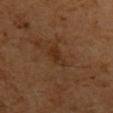The lesion was tiled from a total-body skin photograph and was not biopsied.
A 15 mm close-up tile from a total-body photography series done for melanoma screening.
The lesion is located on the arm.
A male patient, aged approximately 60.
About 3 mm across.
An algorithmic analysis of the crop reported a footprint of about 4.5 mm² and a symmetry-axis asymmetry near 0.35. The software also gave a border-irregularity index near 3.5/10, a within-lesion color-variation index near 3/10, and a peripheral color-asymmetry measure near 1. And it measured a classifier nevus-likeness of about 0/100.
Imaged with cross-polarized lighting.